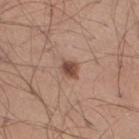Findings:
- location — the right thigh
- image source — ~15 mm crop, total-body skin-cancer survey
- illumination — white-light illumination
- patient — male, aged 38–42